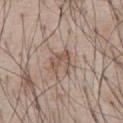Part of a total-body skin-imaging series; this lesion was reviewed on a skin check and was not flagged for biopsy. About 3 mm across. The tile uses white-light illumination. On the chest. A 15 mm close-up tile from a total-body photography series done for melanoma screening. A male patient, about 70 years old.